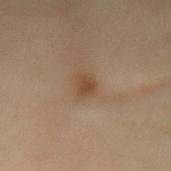tile lighting = cross-polarized illumination
lesion size = ≈3 mm
image source = 15 mm crop, total-body photography
subject = female, about 60 years old
site = the mid back